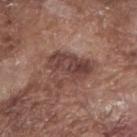The lesion was tiled from a total-body skin photograph and was not biopsied. The lesion is located on the right forearm. This is a white-light tile. A male patient, approximately 75 years of age. Approximately 5 mm at its widest. A lesion tile, about 15 mm wide, cut from a 3D total-body photograph.